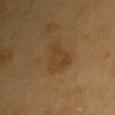  biopsy_status: not biopsied; imaged during a skin examination
  lighting: cross-polarized
  patient:
    sex: male
    age_approx: 60
  lesion_size:
    long_diameter_mm_approx: 4.5
  site: chest
  image:
    source: total-body photography crop
    field_of_view_mm: 15